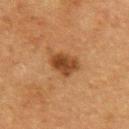{
  "biopsy_status": "not biopsied; imaged during a skin examination",
  "image": {
    "source": "total-body photography crop",
    "field_of_view_mm": 15
  },
  "patient": {
    "sex": "male",
    "age_approx": 75
  },
  "lighting": "cross-polarized",
  "site": "upper back",
  "lesion_size": {
    "long_diameter_mm_approx": 4.0
  }
}